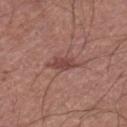workup: imaged on a skin check; not biopsied
illumination: white-light illumination
automated lesion analysis: a footprint of about 5.5 mm², an outline eccentricity of about 0.85 (0 = round, 1 = elongated), and two-axis asymmetry of about 0.35; a mean CIELAB color near L≈45 a*≈23 b*≈23
location: the left thigh
size: about 4 mm
image: ~15 mm tile from a whole-body skin photo
patient: male, in their mid-60s